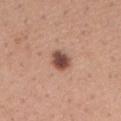This lesion was catalogued during total-body skin photography and was not selected for biopsy.
Longest diameter approximately 3 mm.
The lesion is on the right forearm.
Cropped from a total-body skin-imaging series; the visible field is about 15 mm.
Imaged with white-light lighting.
The subject is a female aged around 30.